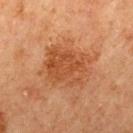The lesion was photographed on a routine skin check and not biopsied; there is no pathology result. A male patient aged around 65. A 15 mm crop from a total-body photograph taken for skin-cancer surveillance. The lesion's longest dimension is about 5 mm. Located on the mid back.